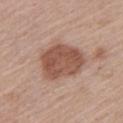This lesion was catalogued during total-body skin photography and was not selected for biopsy. This is a white-light tile. A lesion tile, about 15 mm wide, cut from a 3D total-body photograph. Located on the left upper arm. Measured at roughly 5.5 mm in maximum diameter. A male subject, aged approximately 65. The lesion-visualizer software estimated a footprint of about 20 mm², a shape eccentricity near 0.6, and a shape-asymmetry score of about 0.15 (0 = symmetric). The software also gave a border-irregularity rating of about 1.5/10, internal color variation of about 3.5 on a 0–10 scale, and radial color variation of about 1. The analysis additionally found a nevus-likeness score of about 55/100 and lesion-presence confidence of about 100/100.